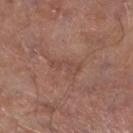size: ≈3.5 mm
image: ~15 mm tile from a whole-body skin photo
tile lighting: white-light illumination
patient: male, approximately 65 years of age
location: the left lower leg